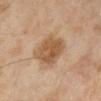{
  "automated_metrics": {
    "cielab_L": 56,
    "cielab_a": 19,
    "cielab_b": 35,
    "vs_skin_darker_L": 11.0,
    "vs_skin_contrast_norm": 8.0,
    "border_irregularity_0_10": 1.5,
    "color_variation_0_10": 4.0,
    "peripheral_color_asymmetry": 1.5
  },
  "patient": {
    "sex": "female",
    "age_approx": 70
  },
  "image": {
    "source": "total-body photography crop",
    "field_of_view_mm": 15
  },
  "site": "right lower leg"
}